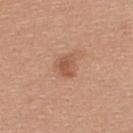About 2.5 mm across. Located on the upper back. An algorithmic analysis of the crop reported an automated nevus-likeness rating near 90 out of 100 and a lesion-detection confidence of about 100/100. This image is a 15 mm lesion crop taken from a total-body photograph. Captured under white-light illumination. A female subject, approximately 20 years of age.